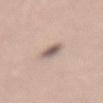The lesion was photographed on a routine skin check and not biopsied; there is no pathology result.